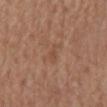Part of a total-body skin-imaging series; this lesion was reviewed on a skin check and was not flagged for biopsy. A lesion tile, about 15 mm wide, cut from a 3D total-body photograph. Captured under white-light illumination. Approximately 2.5 mm at its widest. The lesion is located on the back. The patient is a male in their mid-60s.